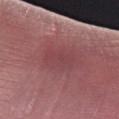  image:
    source: total-body photography crop
    field_of_view_mm: 15
  site: left forearm
  lighting: white-light
  patient:
    sex: male
    age_approx: 70
  automated_metrics:
    area_mm2_approx: 9.0
    shape_asymmetry: 0.15
    border_irregularity_0_10: 2.0
    color_variation_0_10: 1.5
    peripheral_color_asymmetry: 0.5